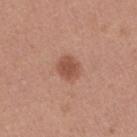Impression: Imaged during a routine full-body skin examination; the lesion was not biopsied and no histopathology is available. Image and clinical context: A female patient, aged 38 to 42. A 15 mm close-up tile from a total-body photography series done for melanoma screening. The lesion is on the right thigh.